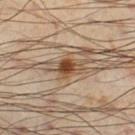biopsy status: no biopsy performed (imaged during a skin exam) | image source: ~15 mm crop, total-body skin-cancer survey | location: the leg | patient: male, in their mid- to late 50s.